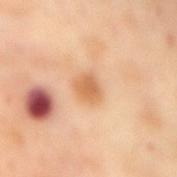Impression: Part of a total-body skin-imaging series; this lesion was reviewed on a skin check and was not flagged for biopsy. Background: About 2.5 mm across. A female subject, in their mid-50s. Imaged with cross-polarized lighting. A 15 mm close-up tile from a total-body photography series done for melanoma screening. The lesion is located on the mid back.